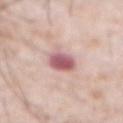Recorded during total-body skin imaging; not selected for excision or biopsy.
A lesion tile, about 15 mm wide, cut from a 3D total-body photograph.
The lesion is located on the front of the torso.
A male patient, roughly 80 years of age.